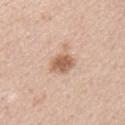biopsy status — no biopsy performed (imaged during a skin exam)
body site — the chest
image source — ~15 mm tile from a whole-body skin photo
image-analysis metrics — a footprint of about 7.5 mm², an eccentricity of roughly 0.65, and two-axis asymmetry of about 0.4; an average lesion color of about L≈62 a*≈19 b*≈31 (CIELAB), roughly 12 lightness units darker than nearby skin, and a normalized lesion–skin contrast near 8; internal color variation of about 3.5 on a 0–10 scale and a peripheral color-asymmetry measure near 1; lesion-presence confidence of about 100/100
subject — male, about 50 years old
lesion size — ≈4 mm